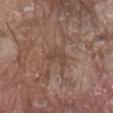  biopsy_status: not biopsied; imaged during a skin examination
  site: abdomen
  image:
    source: total-body photography crop
    field_of_view_mm: 15
  patient:
    sex: male
    age_approx: 80
  lesion_size:
    long_diameter_mm_approx: 3.0